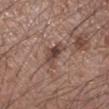Clinical impression:
Recorded during total-body skin imaging; not selected for excision or biopsy.
Clinical summary:
Measured at roughly 3 mm in maximum diameter. This image is a 15 mm lesion crop taken from a total-body photograph. A male subject, aged around 55. The lesion is on the left forearm. Captured under white-light illumination.